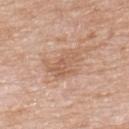biopsy status = no biopsy performed (imaged during a skin exam)
TBP lesion metrics = a footprint of about 8.5 mm², a shape eccentricity near 0.8, and a symmetry-axis asymmetry near 0.4; a lesion color around L≈59 a*≈20 b*≈31 in CIELAB and a lesion–skin lightness drop of about 8; border irregularity of about 6 on a 0–10 scale, a color-variation rating of about 3/10, and a peripheral color-asymmetry measure near 1; lesion-presence confidence of about 100/100
illumination = white-light illumination
image = 15 mm crop, total-body photography
body site = the back
subject = female, roughly 75 years of age